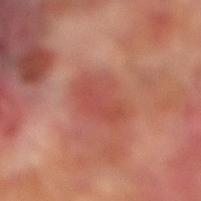Imaged during a routine full-body skin examination; the lesion was not biopsied and no histopathology is available. A male subject aged 68–72. About 5 mm across. From the right lower leg. A 15 mm crop from a total-body photograph taken for skin-cancer surveillance. This is a cross-polarized tile.